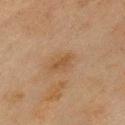Part of a total-body skin-imaging series; this lesion was reviewed on a skin check and was not flagged for biopsy. Cropped from a total-body skin-imaging series; the visible field is about 15 mm. The lesion's longest dimension is about 2.5 mm. Automated tile analysis of the lesion measured a lesion area of about 2.5 mm², an eccentricity of roughly 0.9, and two-axis asymmetry of about 0.35. The software also gave a lesion color around L≈40 a*≈16 b*≈31 in CIELAB, a lesion–skin lightness drop of about 6, and a normalized lesion–skin contrast near 6.5. It also reported a border-irregularity rating of about 4/10 and a peripheral color-asymmetry measure near 0. It also reported an automated nevus-likeness rating near 5 out of 100 and lesion-presence confidence of about 100/100. Located on the chest. The tile uses cross-polarized illumination. The subject is a male in their mid-40s.